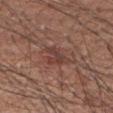| field | value |
|---|---|
| biopsy status | catalogued during a skin exam; not biopsied |
| imaging modality | ~15 mm tile from a whole-body skin photo |
| anatomic site | the right upper arm |
| subject | male, aged around 60 |